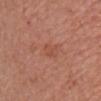{"biopsy_status": "not biopsied; imaged during a skin examination", "image": {"source": "total-body photography crop", "field_of_view_mm": 15}, "site": "chest", "patient": {"sex": "female", "age_approx": 55}, "lighting": "white-light", "lesion_size": {"long_diameter_mm_approx": 3.0}, "automated_metrics": {"area_mm2_approx": 3.5, "eccentricity": 0.8, "shape_asymmetry": 0.25, "cielab_L": 51, "cielab_a": 25, "cielab_b": 31, "vs_skin_darker_L": 5.0, "vs_skin_contrast_norm": 4.5, "color_variation_0_10": 1.5, "peripheral_color_asymmetry": 0.5, "nevus_likeness_0_100": 0, "lesion_detection_confidence_0_100": 100}}